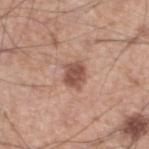Captured during whole-body skin photography for melanoma surveillance; the lesion was not biopsied.